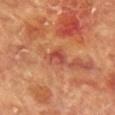Part of a total-body skin-imaging series; this lesion was reviewed on a skin check and was not flagged for biopsy.
From the chest.
The recorded lesion diameter is about 2.5 mm.
An algorithmic analysis of the crop reported an average lesion color of about L≈45 a*≈29 b*≈30 (CIELAB), roughly 8 lightness units darker than nearby skin, and a normalized border contrast of about 7. And it measured radial color variation of about 1.5.
A roughly 15 mm field-of-view crop from a total-body skin photograph.
This is a cross-polarized tile.
The patient is a female about 80 years old.